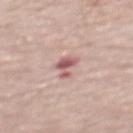Clinical impression: Part of a total-body skin-imaging series; this lesion was reviewed on a skin check and was not flagged for biopsy. Context: The lesion is on the back. A region of skin cropped from a whole-body photographic capture, roughly 15 mm wide. This is a white-light tile. A male patient aged around 70. The recorded lesion diameter is about 3 mm. An algorithmic analysis of the crop reported a border-irregularity index near 6/10 and a peripheral color-asymmetry measure near 1. And it measured an automated nevus-likeness rating near 0 out of 100 and a lesion-detection confidence of about 100/100.